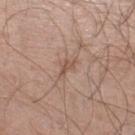Recorded during total-body skin imaging; not selected for excision or biopsy. An algorithmic analysis of the crop reported peripheral color asymmetry of about 0. It also reported an automated nevus-likeness rating near 0 out of 100 and a lesion-detection confidence of about 70/100. A male patient, about 60 years old. About 3 mm across. A 15 mm crop from a total-body photograph taken for skin-cancer surveillance. Located on the left lower leg.